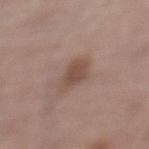{
  "biopsy_status": "not biopsied; imaged during a skin examination",
  "lesion_size": {
    "long_diameter_mm_approx": 3.0
  },
  "patient": {
    "sex": "female",
    "age_approx": 65
  },
  "automated_metrics": {
    "cielab_L": 49,
    "cielab_a": 17,
    "cielab_b": 24,
    "vs_skin_darker_L": 9.0,
    "nevus_likeness_0_100": 70,
    "lesion_detection_confidence_0_100": 100
  },
  "image": {
    "source": "total-body photography crop",
    "field_of_view_mm": 15
  },
  "site": "left lower leg",
  "lighting": "white-light"
}